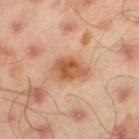Case summary:
* follow-up · total-body-photography surveillance lesion; no biopsy
* acquisition · ~15 mm tile from a whole-body skin photo
* location · the left thigh
* lesion diameter · ≈4 mm
* lighting · cross-polarized
* subject · male, aged approximately 45